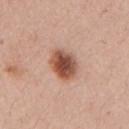lighting: white-light
site: right upper arm
lesion_size:
  long_diameter_mm_approx: 4.0
image:
  source: total-body photography crop
  field_of_view_mm: 15
patient:
  sex: female
  age_approx: 45